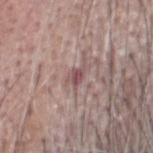Notes:
• biopsy status — imaged on a skin check; not biopsied
• body site — the head or neck
• illumination — white-light
• imaging modality — total-body-photography crop, ~15 mm field of view
• patient — male, approximately 70 years of age